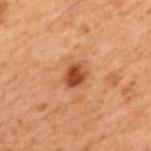Cropped from a total-body skin-imaging series; the visible field is about 15 mm. From the upper back. The patient is a male roughly 70 years of age.